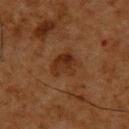Clinical impression: Captured during whole-body skin photography for melanoma surveillance; the lesion was not biopsied. Image and clinical context: A roughly 15 mm field-of-view crop from a total-body skin photograph. A male subject roughly 60 years of age. Located on the upper back. Longest diameter approximately 3.5 mm. Imaged with cross-polarized lighting.